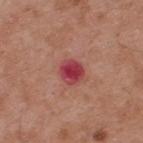image-analysis metrics = an average lesion color of about L≈43 a*≈38 b*≈22 (CIELAB), a lesion–skin lightness drop of about 13, and a normalized lesion–skin contrast near 10.5; border irregularity of about 1.5 on a 0–10 scale, internal color variation of about 4.5 on a 0–10 scale, and radial color variation of about 1.5
illumination = white-light
diameter = ≈2.5 mm
patient = male, aged 53 to 57
image source = ~15 mm crop, total-body skin-cancer survey
location = the upper back